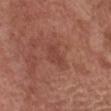Recorded during total-body skin imaging; not selected for excision or biopsy.
Imaged with white-light lighting.
Measured at roughly 3 mm in maximum diameter.
A close-up tile cropped from a whole-body skin photograph, about 15 mm across.
A male subject, aged 63–67.
Located on the chest.